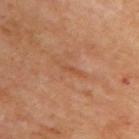<tbp_lesion>
<biopsy_status>not biopsied; imaged during a skin examination</biopsy_status>
<patient>
  <sex>male</sex>
  <age_approx>70</age_approx>
</patient>
<site>upper back</site>
<image>
  <source>total-body photography crop</source>
  <field_of_view_mm>15</field_of_view_mm>
</image>
</tbp_lesion>